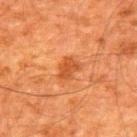Clinical impression:
The lesion was photographed on a routine skin check and not biopsied; there is no pathology result.
Image and clinical context:
Imaged with cross-polarized lighting. Cropped from a whole-body photographic skin survey; the tile spans about 15 mm. A male subject, about 60 years old. The lesion is located on the upper back. The lesion's longest dimension is about 2.5 mm.